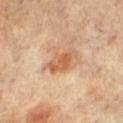Clinical impression:
Imaged during a routine full-body skin examination; the lesion was not biopsied and no histopathology is available.
Context:
Located on the right leg. Imaged with cross-polarized lighting. A 15 mm close-up extracted from a 3D total-body photography capture. The recorded lesion diameter is about 4 mm. A female subject, aged around 65. Automated tile analysis of the lesion measured a mean CIELAB color near L≈58 a*≈22 b*≈37 and a normalized lesion–skin contrast near 8.